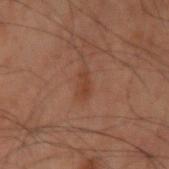The lesion was photographed on a routine skin check and not biopsied; there is no pathology result. The patient is a male aged approximately 60. The lesion's longest dimension is about 2.5 mm. Located on the arm. A 15 mm close-up tile from a total-body photography series done for melanoma screening. Captured under cross-polarized illumination.